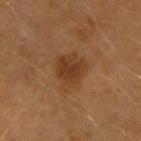Q: Was this lesion biopsied?
A: no biopsy performed (imaged during a skin exam)
Q: What are the patient's age and sex?
A: male, approximately 40 years of age
Q: Lesion location?
A: the right forearm
Q: What is the imaging modality?
A: ~15 mm crop, total-body skin-cancer survey
Q: What is the lesion's diameter?
A: about 4.5 mm